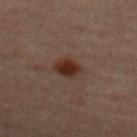image-analysis metrics = border irregularity of about 2 on a 0–10 scale, a within-lesion color-variation index near 3/10, and a peripheral color-asymmetry measure near 1; a nevus-likeness score of about 95/100 and lesion-presence confidence of about 100/100
lighting = cross-polarized
site = the abdomen
lesion diameter = ~3 mm (longest diameter)
patient = male, aged approximately 60
imaging modality = ~15 mm tile from a whole-body skin photo